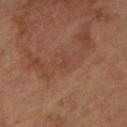The lesion is on the left lower leg. Longest diameter approximately 1.5 mm. A 15 mm close-up tile from a total-body photography series done for melanoma screening. A female patient in their mid- to late 60s. Imaged with cross-polarized lighting. The total-body-photography lesion software estimated an automated nevus-likeness rating near 0 out of 100.